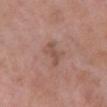Impression: No biopsy was performed on this lesion — it was imaged during a full skin examination and was not determined to be concerning. Context: From the chest. About 3.5 mm across. A female patient, aged approximately 70. The tile uses white-light illumination. A close-up tile cropped from a whole-body skin photograph, about 15 mm across.